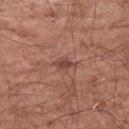follow-up = catalogued during a skin exam; not biopsied
illumination = white-light
image source = ~15 mm crop, total-body skin-cancer survey
lesion size = ≈3 mm
automated lesion analysis = a lesion area of about 3 mm², an eccentricity of roughly 0.8, and a symmetry-axis asymmetry near 0.3; an average lesion color of about L≈45 a*≈23 b*≈25 (CIELAB), about 9 CIELAB-L* units darker than the surrounding skin, and a normalized lesion–skin contrast near 7
subject = male, aged around 65
anatomic site = the left upper arm